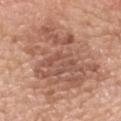Image and clinical context: The patient is a male aged approximately 50. On the upper back. A 15 mm crop from a total-body photograph taken for skin-cancer surveillance. This is a white-light tile. About 11.5 mm across. Conclusion: On biopsy, histopathology showed a melanoma in situ, classified as a malignant skin lesion.